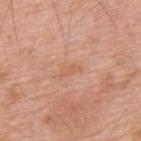• follow-up — total-body-photography surveillance lesion; no biopsy
• image — ~15 mm tile from a whole-body skin photo
• image-analysis metrics — a mean CIELAB color near L≈61 a*≈22 b*≈34, about 5 CIELAB-L* units darker than the surrounding skin, and a lesion-to-skin contrast of about 4.5 (normalized; higher = more distinct); a border-irregularity index near 4/10, internal color variation of about 0 on a 0–10 scale, and peripheral color asymmetry of about 0; a nevus-likeness score of about 0/100 and a detector confidence of about 100 out of 100 that the crop contains a lesion
• lesion diameter — about 3 mm
• body site — the back
• illumination — white-light illumination
• patient — male, roughly 60 years of age